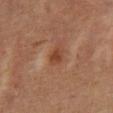biopsy status: imaged on a skin check; not biopsied
imaging modality: ~15 mm crop, total-body skin-cancer survey
lighting: cross-polarized
patient: male, approximately 60 years of age
anatomic site: the mid back
diameter: about 2.5 mm